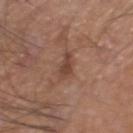Q: Was a biopsy performed?
A: no biopsy performed (imaged during a skin exam)
Q: How was this image acquired?
A: ~15 mm tile from a whole-body skin photo
Q: What are the patient's age and sex?
A: male, roughly 60 years of age
Q: Where on the body is the lesion?
A: the head or neck